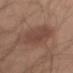Assessment: Part of a total-body skin-imaging series; this lesion was reviewed on a skin check and was not flagged for biopsy. Context: The tile uses white-light illumination. Measured at roughly 6 mm in maximum diameter. Located on the left upper arm. An algorithmic analysis of the crop reported a mean CIELAB color near L≈45 a*≈19 b*≈25, a lesion–skin lightness drop of about 8, and a normalized border contrast of about 6.5. It also reported border irregularity of about 3 on a 0–10 scale, a within-lesion color-variation index near 2.5/10, and a peripheral color-asymmetry measure near 1. A male subject, aged 43 to 47. Cropped from a whole-body photographic skin survey; the tile spans about 15 mm.